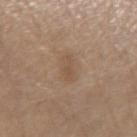Assessment: Part of a total-body skin-imaging series; this lesion was reviewed on a skin check and was not flagged for biopsy. Context: A male subject roughly 65 years of age. A lesion tile, about 15 mm wide, cut from a 3D total-body photograph. Imaged with white-light lighting. From the left forearm. Measured at roughly 3.5 mm in maximum diameter.